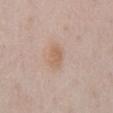Clinical impression:
No biopsy was performed on this lesion — it was imaged during a full skin examination and was not determined to be concerning.
Image and clinical context:
On the abdomen. A 15 mm close-up extracted from a 3D total-body photography capture. Approximately 3 mm at its widest. A male patient about 60 years old. This is a white-light tile.